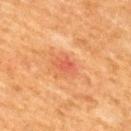This lesion was catalogued during total-body skin photography and was not selected for biopsy.
Located on the back.
A male patient, about 50 years old.
Measured at roughly 2.5 mm in maximum diameter.
Captured under cross-polarized illumination.
A 15 mm close-up tile from a total-body photography series done for melanoma screening.